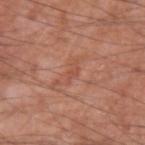Captured during whole-body skin photography for melanoma surveillance; the lesion was not biopsied.
A roughly 15 mm field-of-view crop from a total-body skin photograph.
Longest diameter approximately 3 mm.
A male patient in their mid- to late 70s.
Automated image analysis of the tile measured a shape eccentricity near 0.9 and two-axis asymmetry of about 0.35. And it measured a mean CIELAB color near L≈52 a*≈26 b*≈31, a lesion–skin lightness drop of about 6, and a normalized border contrast of about 4.5. The analysis additionally found a classifier nevus-likeness of about 0/100 and a lesion-detection confidence of about 95/100.
This is a white-light tile.
Located on the left upper arm.